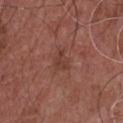notes: no biopsy performed (imaged during a skin exam)
diameter: ~2.5 mm (longest diameter)
tile lighting: white-light
body site: the chest
subject: male, approximately 65 years of age
imaging modality: 15 mm crop, total-body photography